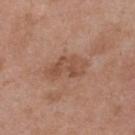{"biopsy_status": "not biopsied; imaged during a skin examination", "automated_metrics": {"area_mm2_approx": 9.5, "eccentricity": 0.85, "shape_asymmetry": 0.3, "cielab_L": 51, "cielab_a": 21, "cielab_b": 30, "vs_skin_darker_L": 8.0, "vs_skin_contrast_norm": 6.0}, "image": {"source": "total-body photography crop", "field_of_view_mm": 15}, "patient": {"sex": "female", "age_approx": 40}, "lesion_size": {"long_diameter_mm_approx": 5.0}, "site": "upper back", "lighting": "white-light"}